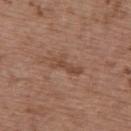| key | value |
|---|---|
| workup | no biopsy performed (imaged during a skin exam) |
| automated metrics | an area of roughly 5 mm², an outline eccentricity of about 0.95 (0 = round, 1 = elongated), and a symmetry-axis asymmetry near 0.4; a border-irregularity rating of about 5.5/10, a color-variation rating of about 1.5/10, and a peripheral color-asymmetry measure near 0 |
| body site | the back |
| diameter | ≈4.5 mm |
| acquisition | total-body-photography crop, ~15 mm field of view |
| patient | female, roughly 65 years of age |
| tile lighting | white-light |